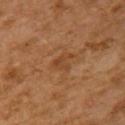Findings:
– notes: total-body-photography surveillance lesion; no biopsy
– image: 15 mm crop, total-body photography
– site: the upper back
– image-analysis metrics: an area of roughly 4 mm², a shape eccentricity near 0.65, and a symmetry-axis asymmetry near 0.5; an average lesion color of about L≈38 a*≈20 b*≈32 (CIELAB), about 6 CIELAB-L* units darker than the surrounding skin, and a normalized lesion–skin contrast near 5.5; a peripheral color-asymmetry measure near 0.5
– illumination: cross-polarized illumination
– patient: female, aged 58 to 62
– diameter: ≈2.5 mm